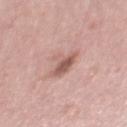– subject · female, in their mid- to late 50s
– illumination · white-light
– lesion diameter · ~3.5 mm (longest diameter)
– image source · ~15 mm crop, total-body skin-cancer survey
– location · the right thigh